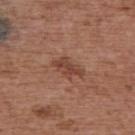The lesion was tiled from a total-body skin photograph and was not biopsied.
A female subject roughly 65 years of age.
Cropped from a whole-body photographic skin survey; the tile spans about 15 mm.
From the upper back.